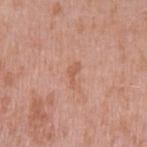Part of a total-body skin-imaging series; this lesion was reviewed on a skin check and was not flagged for biopsy. Automated tile analysis of the lesion measured two-axis asymmetry of about 0.45. It also reported a mean CIELAB color near L≈58 a*≈24 b*≈32, about 7 CIELAB-L* units darker than the surrounding skin, and a normalized border contrast of about 5.5. And it measured border irregularity of about 5 on a 0–10 scale, a within-lesion color-variation index near 0/10, and a peripheral color-asymmetry measure near 0. A male patient, about 40 years old. The tile uses white-light illumination. Located on the arm. A close-up tile cropped from a whole-body skin photograph, about 15 mm across. The recorded lesion diameter is about 2.5 mm.